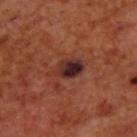Assessment: The lesion was tiled from a total-body skin photograph and was not biopsied. Image and clinical context: Measured at roughly 4 mm in maximum diameter. A region of skin cropped from a whole-body photographic capture, roughly 15 mm wide. The subject is a male aged 68 to 72. The tile uses cross-polarized illumination. The lesion is on the back.